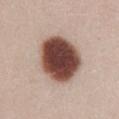The subject is a female about 25 years old. Automated image analysis of the tile measured an area of roughly 26 mm², an outline eccentricity of about 0.5 (0 = round, 1 = elongated), and two-axis asymmetry of about 0.1. Cropped from a total-body skin-imaging series; the visible field is about 15 mm. The recorded lesion diameter is about 6 mm. The lesion is located on the abdomen. The biopsy diagnosis was a benign skin lesion: dysplastic (Clark) nevus.